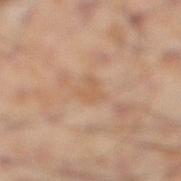biopsy_status: not biopsied; imaged during a skin examination
lighting: cross-polarized
image:
  source: total-body photography crop
  field_of_view_mm: 15
automated_metrics:
  color_variation_0_10: 1.5
  peripheral_color_asymmetry: 0.5
patient:
  sex: male
  age_approx: 55
lesion_size:
  long_diameter_mm_approx: 3.0
site: right lower leg